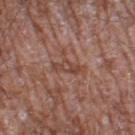Notes:
- notes — catalogued during a skin exam; not biopsied
- patient — male, aged 58 to 62
- site — the left thigh
- automated metrics — a lesion area of about 4 mm², an outline eccentricity of about 0.9 (0 = round, 1 = elongated), and a shape-asymmetry score of about 0.35 (0 = symmetric); border irregularity of about 4.5 on a 0–10 scale, a color-variation rating of about 1/10, and a peripheral color-asymmetry measure near 0.5; lesion-presence confidence of about 70/100
- size — ≈3.5 mm
- image — ~15 mm tile from a whole-body skin photo